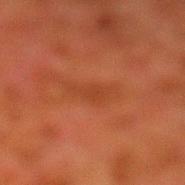Clinical impression:
No biopsy was performed on this lesion — it was imaged during a full skin examination and was not determined to be concerning.
Acquisition and patient details:
A 15 mm close-up tile from a total-body photography series done for melanoma screening. A male subject approximately 80 years of age. On the left lower leg.